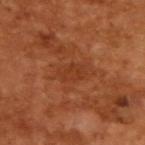Assessment:
This lesion was catalogued during total-body skin photography and was not selected for biopsy.
Context:
Longest diameter approximately 3.5 mm. A male subject aged 63–67. Imaged with cross-polarized lighting. The lesion-visualizer software estimated a lesion color around L≈37 a*≈27 b*≈35 in CIELAB, about 5 CIELAB-L* units darker than the surrounding skin, and a normalized lesion–skin contrast near 5. And it measured a border-irregularity rating of about 2.5/10 and radial color variation of about 1. The analysis additionally found a nevus-likeness score of about 0/100 and a lesion-detection confidence of about 100/100. This image is a 15 mm lesion crop taken from a total-body photograph.